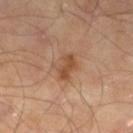notes = no biopsy performed (imaged during a skin exam); patient = male, aged approximately 65; image = 15 mm crop, total-body photography; size = ≈3.5 mm; location = the left lower leg.